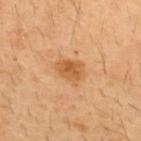biopsy status = catalogued during a skin exam; not biopsied
body site = the upper back
lesion diameter = about 3.5 mm
acquisition = 15 mm crop, total-body photography
TBP lesion metrics = an area of roughly 7 mm²; a border-irregularity rating of about 2.5/10 and radial color variation of about 1
subject = male, aged approximately 30